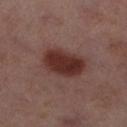Imaged during a routine full-body skin examination; the lesion was not biopsied and no histopathology is available. A 15 mm crop from a total-body photograph taken for skin-cancer surveillance. Approximately 5.5 mm at its widest. A female patient, aged 53 to 57. The lesion is on the right lower leg.